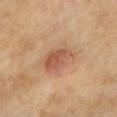This lesion was catalogued during total-body skin photography and was not selected for biopsy. This is a cross-polarized tile. Located on the chest. The recorded lesion diameter is about 4.5 mm. The patient is a male in their 70s. Cropped from a whole-body photographic skin survey; the tile spans about 15 mm.